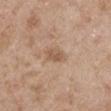workup: no biopsy performed (imaged during a skin exam) | automated lesion analysis: internal color variation of about 2 on a 0–10 scale and a peripheral color-asymmetry measure near 1 | lighting: white-light | subject: male, in their 50s | body site: the right upper arm | diameter: about 2.5 mm | image source: ~15 mm crop, total-body skin-cancer survey.